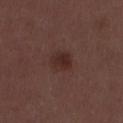Context: A 15 mm crop from a total-body photograph taken for skin-cancer surveillance. The lesion is on the mid back. A male subject aged around 30.